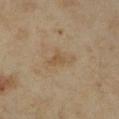Impression:
The lesion was photographed on a routine skin check and not biopsied; there is no pathology result.
Clinical summary:
A close-up tile cropped from a whole-body skin photograph, about 15 mm across. The lesion is on the right upper arm. The recorded lesion diameter is about 4 mm. A female patient approximately 35 years of age. The tile uses cross-polarized illumination.